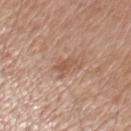Imaged during a routine full-body skin examination; the lesion was not biopsied and no histopathology is available. This image is a 15 mm lesion crop taken from a total-body photograph. Imaged with white-light lighting. The lesion is located on the left upper arm. A male subject aged 68–72. The recorded lesion diameter is about 3 mm.